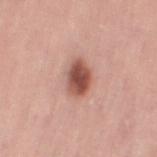The lesion was photographed on a routine skin check and not biopsied; there is no pathology result. Located on the left thigh. A 15 mm close-up extracted from a 3D total-body photography capture. A female patient aged 48 to 52.